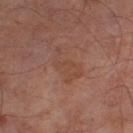workup = catalogued during a skin exam; not biopsied | lesion size = ≈4 mm | subject = male, in their mid-60s | image source = total-body-photography crop, ~15 mm field of view | body site = the left thigh.